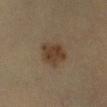Imaged during a routine full-body skin examination; the lesion was not biopsied and no histopathology is available. Imaged with cross-polarized lighting. The total-body-photography lesion software estimated an area of roughly 9.5 mm² and an outline eccentricity of about 0.7 (0 = round, 1 = elongated). The analysis additionally found a mean CIELAB color near L≈34 a*≈13 b*≈25 and a lesion–skin lightness drop of about 8. The lesion is located on the left lower leg. A 15 mm close-up extracted from a 3D total-body photography capture. A female subject, in their 30s.